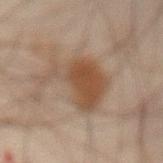biopsy_status: not biopsied; imaged during a skin examination
image:
  source: total-body photography crop
  field_of_view_mm: 15
site: abdomen
lighting: cross-polarized
lesion_size:
  long_diameter_mm_approx: 7.0
patient:
  sex: male
  age_approx: 45